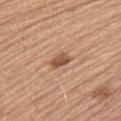Captured during whole-body skin photography for melanoma surveillance; the lesion was not biopsied.
Automated tile analysis of the lesion measured an outline eccentricity of about 0.7 (0 = round, 1 = elongated) and a symmetry-axis asymmetry near 0.15. The analysis additionally found a within-lesion color-variation index near 3.5/10 and a peripheral color-asymmetry measure near 1.
The lesion is on the arm.
A close-up tile cropped from a whole-body skin photograph, about 15 mm across.
Approximately 2.5 mm at its widest.
A male patient roughly 55 years of age.